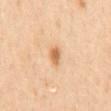Case summary:
- workup — catalogued during a skin exam; not biopsied
- illumination — cross-polarized illumination
- lesion diameter — about 2.5 mm
- image source — ~15 mm crop, total-body skin-cancer survey
- site — the mid back
- subject — male, aged approximately 65